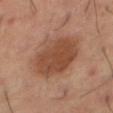A male subject, aged approximately 65. From the leg. Cropped from a whole-body photographic skin survey; the tile spans about 15 mm. Approximately 7 mm at its widest. This is a cross-polarized tile.